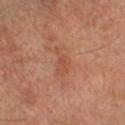Recorded during total-body skin imaging; not selected for excision or biopsy.
Longest diameter approximately 3.5 mm.
A male patient, roughly 60 years of age.
Captured under cross-polarized illumination.
The lesion is located on the right lower leg.
The lesion-visualizer software estimated a lesion area of about 4.5 mm², an eccentricity of roughly 0.9, and a symmetry-axis asymmetry near 0.55. And it measured a lesion color around L≈46 a*≈24 b*≈29 in CIELAB, about 6 CIELAB-L* units darker than the surrounding skin, and a normalized lesion–skin contrast near 4.5. It also reported an automated nevus-likeness rating near 0 out of 100 and a lesion-detection confidence of about 100/100.
A 15 mm crop from a total-body photograph taken for skin-cancer surveillance.